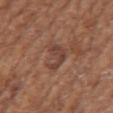Recorded during total-body skin imaging; not selected for excision or biopsy. The lesion is on the left upper arm. The patient is a female aged around 75. Cropped from a whole-body photographic skin survey; the tile spans about 15 mm. This is a white-light tile. The total-body-photography lesion software estimated an area of roughly 6.5 mm², an outline eccentricity of about 0.8 (0 = round, 1 = elongated), and a symmetry-axis asymmetry near 0.45. And it measured a lesion–skin lightness drop of about 8. It also reported a border-irregularity rating of about 6/10. The software also gave a classifier nevus-likeness of about 0/100 and lesion-presence confidence of about 95/100. The recorded lesion diameter is about 4 mm.